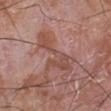No biopsy was performed on this lesion — it was imaged during a full skin examination and was not determined to be concerning.
Located on the right lower leg.
The tile uses white-light illumination.
Automated image analysis of the tile measured a border-irregularity index near 9.5/10, a within-lesion color-variation index near 3/10, and peripheral color asymmetry of about 1. It also reported an automated nevus-likeness rating near 0 out of 100.
A male subject aged 58–62.
This image is a 15 mm lesion crop taken from a total-body photograph.
The recorded lesion diameter is about 7 mm.